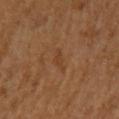An algorithmic analysis of the crop reported an area of roughly 3 mm² and two-axis asymmetry of about 0.3. The software also gave a lesion color around L≈39 a*≈20 b*≈33 in CIELAB and a lesion-to-skin contrast of about 4.5 (normalized; higher = more distinct). It also reported border irregularity of about 3 on a 0–10 scale, a within-lesion color-variation index near 0.5/10, and peripheral color asymmetry of about 0. The analysis additionally found an automated nevus-likeness rating near 0 out of 100 and a lesion-detection confidence of about 100/100. Captured under cross-polarized illumination. Cropped from a total-body skin-imaging series; the visible field is about 15 mm. The lesion is on the arm. The patient is a female in their 50s. Measured at roughly 3 mm in maximum diameter.